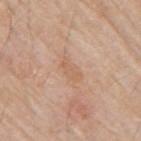Q: Where on the body is the lesion?
A: the right upper arm
Q: What lighting was used for the tile?
A: white-light illumination
Q: Lesion size?
A: ~3 mm (longest diameter)
Q: Who is the patient?
A: male, about 80 years old
Q: What is the imaging modality?
A: total-body-photography crop, ~15 mm field of view